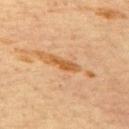Image and clinical context:
On the back. The tile uses cross-polarized illumination. The subject is a male aged 63–67. The total-body-photography lesion software estimated an area of roughly 3.5 mm² and a shape-asymmetry score of about 0.4 (0 = symmetric). The software also gave roughly 10 lightness units darker than nearby skin and a lesion-to-skin contrast of about 8.5 (normalized; higher = more distinct). The analysis additionally found border irregularity of about 5 on a 0–10 scale and a peripheral color-asymmetry measure near 0. The software also gave a classifier nevus-likeness of about 0/100. A 15 mm close-up tile from a total-body photography series done for melanoma screening. The lesion's longest dimension is about 3.5 mm.